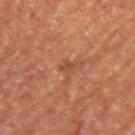Impression: Part of a total-body skin-imaging series; this lesion was reviewed on a skin check and was not flagged for biopsy. Acquisition and patient details: The tile uses cross-polarized illumination. A 15 mm close-up extracted from a 3D total-body photography capture. Automated tile analysis of the lesion measured a shape-asymmetry score of about 0.3 (0 = symmetric). And it measured an average lesion color of about L≈41 a*≈21 b*≈31 (CIELAB), roughly 7 lightness units darker than nearby skin, and a lesion-to-skin contrast of about 6 (normalized; higher = more distinct). And it measured a border-irregularity index near 2.5/10, a within-lesion color-variation index near 0/10, and peripheral color asymmetry of about 0. The analysis additionally found a lesion-detection confidence of about 100/100. A male patient, aged 53 to 57. On the left upper arm. The recorded lesion diameter is about 1.5 mm.